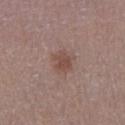| key | value |
|---|---|
| biopsy status | no biopsy performed (imaged during a skin exam) |